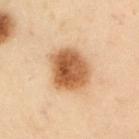Automated tile analysis of the lesion measured a footprint of about 17 mm² and two-axis asymmetry of about 0.1. The analysis additionally found a color-variation rating of about 5.5/10. A male patient aged approximately 55. Cropped from a whole-body photographic skin survey; the tile spans about 15 mm. The lesion is on the left upper arm. The recorded lesion diameter is about 5 mm. Imaged with cross-polarized lighting.